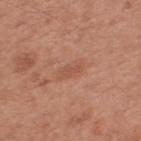Part of a total-body skin-imaging series; this lesion was reviewed on a skin check and was not flagged for biopsy. Captured under white-light illumination. A lesion tile, about 15 mm wide, cut from a 3D total-body photograph. The lesion-visualizer software estimated a footprint of about 2.5 mm² and an outline eccentricity of about 0.9 (0 = round, 1 = elongated). The software also gave a border-irregularity index near 4/10, a within-lesion color-variation index near 0/10, and a peripheral color-asymmetry measure near 0. And it measured an automated nevus-likeness rating near 5 out of 100 and a lesion-detection confidence of about 100/100. The patient is a male aged 53–57. The lesion is located on the upper back. The lesion's longest dimension is about 2.5 mm.